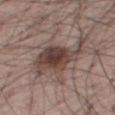{
  "biopsy_status": "not biopsied; imaged during a skin examination",
  "lesion_size": {
    "long_diameter_mm_approx": 7.5
  },
  "patient": {
    "sex": "male",
    "age_approx": 70
  },
  "lighting": "white-light",
  "site": "left leg",
  "image": {
    "source": "total-body photography crop",
    "field_of_view_mm": 15
  }
}